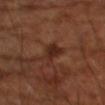anatomic site: the right upper arm; subject: male, approximately 65 years of age; size: ≈3 mm; image: total-body-photography crop, ~15 mm field of view; tile lighting: cross-polarized illumination; automated lesion analysis: a lesion–skin lightness drop of about 8 and a normalized border contrast of about 8.5.